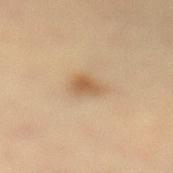<record>
<lesion_size>
  <long_diameter_mm_approx>3.0</long_diameter_mm_approx>
</lesion_size>
<image>
  <source>total-body photography crop</source>
  <field_of_view_mm>15</field_of_view_mm>
</image>
<automated_metrics>
  <cielab_L>53</cielab_L>
  <cielab_a>16</cielab_a>
  <cielab_b>33</cielab_b>
  <vs_skin_darker_L>9.0</vs_skin_darker_L>
  <vs_skin_contrast_norm>7.0</vs_skin_contrast_norm>
  <border_irregularity_0_10>2.5</border_irregularity_0_10>
  <color_variation_0_10>2.5</color_variation_0_10>
  <peripheral_color_asymmetry>0.5</peripheral_color_asymmetry>
  <nevus_likeness_0_100>90</nevus_likeness_0_100>
  <lesion_detection_confidence_0_100>100</lesion_detection_confidence_0_100>
</automated_metrics>
<site>left lower leg</site>
<patient>
  <sex>female</sex>
  <age_approx>30</age_approx>
</patient>
</record>